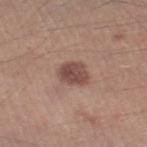workup=total-body-photography surveillance lesion; no biopsy
subject=male, aged 38–42
lesion size=≈3.5 mm
anatomic site=the right lower leg
image source=~15 mm tile from a whole-body skin photo